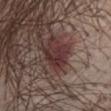{
  "biopsy_status": "not biopsied; imaged during a skin examination",
  "patient": {
    "sex": "male",
    "age_approx": 40
  },
  "lighting": "white-light",
  "image": {
    "source": "total-body photography crop",
    "field_of_view_mm": 15
  },
  "automated_metrics": {
    "area_mm2_approx": 7.0,
    "eccentricity": 0.75,
    "shape_asymmetry": 0.35,
    "nevus_likeness_0_100": 85
  },
  "site": "chest"
}